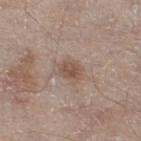notes: total-body-photography surveillance lesion; no biopsy
tile lighting: white-light illumination
site: the right thigh
lesion size: about 2.5 mm
subject: male, in their mid-60s
image source: ~15 mm crop, total-body skin-cancer survey